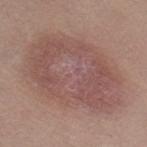Q: Was this lesion biopsied?
A: imaged on a skin check; not biopsied
Q: How was this image acquired?
A: total-body-photography crop, ~15 mm field of view
Q: What did automated image analysis measure?
A: a lesion color around L≈52 a*≈19 b*≈22 in CIELAB, roughly 8 lightness units darker than nearby skin, and a normalized border contrast of about 6; a border-irregularity rating of about 2/10 and peripheral color asymmetry of about 1.5; a nevus-likeness score of about 55/100 and lesion-presence confidence of about 100/100
Q: Illumination type?
A: white-light illumination
Q: What is the lesion's diameter?
A: about 11.5 mm
Q: What are the patient's age and sex?
A: female, aged 33–37
Q: Where on the body is the lesion?
A: the right thigh